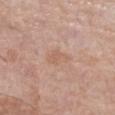biopsy status — no biopsy performed (imaged during a skin exam) | illumination — white-light illumination | image — ~15 mm tile from a whole-body skin photo | subject — female, approximately 70 years of age | automated metrics — an automated nevus-likeness rating near 0 out of 100 | anatomic site — the left lower leg | lesion diameter — about 3.5 mm.